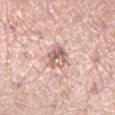The lesion was photographed on a routine skin check and not biopsied; there is no pathology result. About 3.5 mm across. On the left lower leg. A female subject, aged 33 to 37. Imaged with white-light lighting. Automated tile analysis of the lesion measured an area of roughly 7 mm², a shape eccentricity near 0.7, and a symmetry-axis asymmetry near 0.25. A 15 mm close-up extracted from a 3D total-body photography capture.